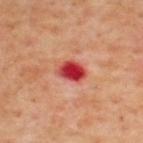Part of a total-body skin-imaging series; this lesion was reviewed on a skin check and was not flagged for biopsy. A male patient roughly 65 years of age. This is a cross-polarized tile. A 15 mm close-up tile from a total-body photography series done for melanoma screening. On the upper back. Longest diameter approximately 3.5 mm.